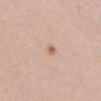Impression:
This lesion was catalogued during total-body skin photography and was not selected for biopsy.
Image and clinical context:
Longest diameter approximately 2.5 mm. The tile uses white-light illumination. Automated tile analysis of the lesion measured a footprint of about 3.5 mm², an outline eccentricity of about 0.75 (0 = round, 1 = elongated), and two-axis asymmetry of about 0.3. And it measured a lesion color around L≈66 a*≈17 b*≈29 in CIELAB, about 7 CIELAB-L* units darker than the surrounding skin, and a normalized lesion–skin contrast near 5. A 15 mm close-up extracted from a 3D total-body photography capture. A female subject in their 40s. The lesion is located on the lower back.